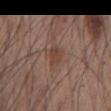biopsy status: total-body-photography surveillance lesion; no biopsy | subject: male, roughly 55 years of age | lighting: white-light | anatomic site: the front of the torso | imaging modality: total-body-photography crop, ~15 mm field of view | diameter: about 3 mm | image-analysis metrics: a lesion area of about 4 mm², an outline eccentricity of about 0.8 (0 = round, 1 = elongated), and two-axis asymmetry of about 0.25; a lesion color around L≈42 a*≈18 b*≈26 in CIELAB and a normalized lesion–skin contrast near 6; a border-irregularity index near 2.5/10, internal color variation of about 2 on a 0–10 scale, and a peripheral color-asymmetry measure near 1; a nevus-likeness score of about 0/100.